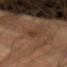Captured during whole-body skin photography for melanoma surveillance; the lesion was not biopsied. The patient is approximately 55 years of age. A region of skin cropped from a whole-body photographic capture, roughly 15 mm wide. Captured under cross-polarized illumination. The lesion is located on the left forearm.